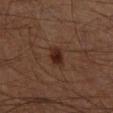Impression:
Captured during whole-body skin photography for melanoma surveillance; the lesion was not biopsied.
Acquisition and patient details:
A male patient, aged 58 to 62. A 15 mm close-up extracted from a 3D total-body photography capture. The lesion is on the right lower leg. About 3 mm across. The total-body-photography lesion software estimated an area of roughly 4.5 mm², an outline eccentricity of about 0.8 (0 = round, 1 = elongated), and a symmetry-axis asymmetry near 0.2. And it measured a lesion color around L≈23 a*≈17 b*≈22 in CIELAB, roughly 8 lightness units darker than nearby skin, and a normalized lesion–skin contrast near 9.5. It also reported a border-irregularity rating of about 2.5/10, a color-variation rating of about 2.5/10, and a peripheral color-asymmetry measure near 1. And it measured a detector confidence of about 100 out of 100 that the crop contains a lesion.